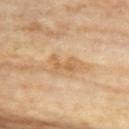<record>
<biopsy_status>not biopsied; imaged during a skin examination</biopsy_status>
<site>chest</site>
<image>
  <source>total-body photography crop</source>
  <field_of_view_mm>15</field_of_view_mm>
</image>
<patient>
  <sex>female</sex>
  <age_approx>75</age_approx>
</patient>
</record>